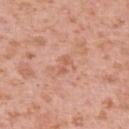A female subject roughly 40 years of age. Located on the left upper arm. A lesion tile, about 15 mm wide, cut from a 3D total-body photograph. Imaged with white-light lighting. An algorithmic analysis of the crop reported a within-lesion color-variation index near 0/10 and radial color variation of about 0. And it measured a nevus-likeness score of about 0/100 and lesion-presence confidence of about 100/100. The recorded lesion diameter is about 2.5 mm.